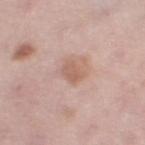{
  "biopsy_status": "not biopsied; imaged during a skin examination",
  "site": "leg",
  "image": {
    "source": "total-body photography crop",
    "field_of_view_mm": 15
  },
  "lighting": "white-light",
  "lesion_size": {
    "long_diameter_mm_approx": 3.0
  },
  "patient": {
    "sex": "female",
    "age_approx": 50
  }
}